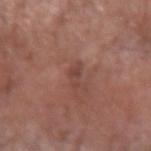Clinical impression:
This lesion was catalogued during total-body skin photography and was not selected for biopsy.